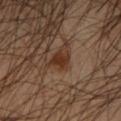Clinical impression: Recorded during total-body skin imaging; not selected for excision or biopsy. Acquisition and patient details: On the right forearm. The patient is a male roughly 45 years of age. Captured under cross-polarized illumination. About 3 mm across. A roughly 15 mm field-of-view crop from a total-body skin photograph.